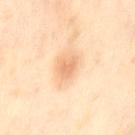Recorded during total-body skin imaging; not selected for excision or biopsy. The lesion is located on the leg. The patient is a female aged 48–52. A roughly 15 mm field-of-view crop from a total-body skin photograph. Captured under cross-polarized illumination.